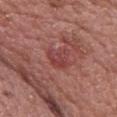  biopsy_status: not biopsied; imaged during a skin examination
  site: upper back
  automated_metrics:
    area_mm2_approx: 6.5
    cielab_L: 43
    cielab_a: 29
    cielab_b: 23
    vs_skin_darker_L: 7.0
    vs_skin_contrast_norm: 5.5
    border_irregularity_0_10: 3.5
    lesion_detection_confidence_0_100: 100
  patient:
    sex: female
    age_approx: 60
  lesion_size:
    long_diameter_mm_approx: 3.0
  lighting: white-light
  image:
    source: total-body photography crop
    field_of_view_mm: 15